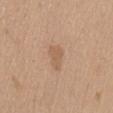Imaged during a routine full-body skin examination; the lesion was not biopsied and no histopathology is available. A 15 mm crop from a total-body photograph taken for skin-cancer surveillance. About 3 mm across. The subject is a male in their 70s. Imaged with white-light lighting. The lesion is located on the lower back. The total-body-photography lesion software estimated a mean CIELAB color near L≈59 a*≈18 b*≈32, about 7 CIELAB-L* units darker than the surrounding skin, and a lesion-to-skin contrast of about 5 (normalized; higher = more distinct). It also reported a border-irregularity index near 3.5/10 and a peripheral color-asymmetry measure near 0.5. The software also gave an automated nevus-likeness rating near 0 out of 100 and a lesion-detection confidence of about 100/100.